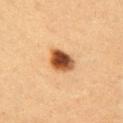This lesion was catalogued during total-body skin photography and was not selected for biopsy. From the right upper arm. About 3.5 mm across. An algorithmic analysis of the crop reported a lesion area of about 7 mm² and two-axis asymmetry of about 0.25. The software also gave a mean CIELAB color near L≈44 a*≈23 b*≈35, roughly 20 lightness units darker than nearby skin, and a normalized border contrast of about 14. The tile uses cross-polarized illumination. The patient is a female aged approximately 40. A lesion tile, about 15 mm wide, cut from a 3D total-body photograph.